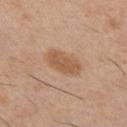<record>
  <biopsy_status>not biopsied; imaged during a skin examination</biopsy_status>
  <patient>
    <sex>male</sex>
    <age_approx>30</age_approx>
  </patient>
  <lighting>white-light</lighting>
  <image>
    <source>total-body photography crop</source>
    <field_of_view_mm>15</field_of_view_mm>
  </image>
  <lesion_size>
    <long_diameter_mm_approx>4.0</long_diameter_mm_approx>
  </lesion_size>
  <site>front of the torso</site>
</record>